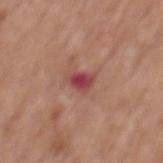Clinical impression: No biopsy was performed on this lesion — it was imaged during a full skin examination and was not determined to be concerning. Acquisition and patient details: The recorded lesion diameter is about 2.5 mm. The tile uses white-light illumination. From the mid back. A 15 mm close-up extracted from a 3D total-body photography capture. A male subject aged approximately 70.